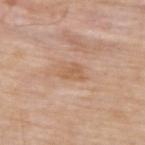<record>
  <biopsy_status>not biopsied; imaged during a skin examination</biopsy_status>
  <lesion_size>
    <long_diameter_mm_approx>3.0</long_diameter_mm_approx>
  </lesion_size>
  <image>
    <source>total-body photography crop</source>
    <field_of_view_mm>15</field_of_view_mm>
  </image>
  <patient>
    <sex>male</sex>
    <age_approx>65</age_approx>
  </patient>
  <lighting>white-light</lighting>
  <site>upper back</site>
</record>